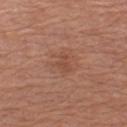follow-up: total-body-photography surveillance lesion; no biopsy